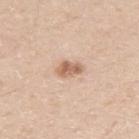Case summary:
– notes — catalogued during a skin exam; not biopsied
– lighting — white-light illumination
– imaging modality — ~15 mm tile from a whole-body skin photo
– patient — male, approximately 30 years of age
– lesion size — about 3 mm
– body site — the left upper arm